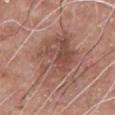  biopsy_status: not biopsied; imaged during a skin examination
  site: front of the torso
  patient:
    sex: male
    age_approx: 60
  lesion_size:
    long_diameter_mm_approx: 8.5
  lighting: white-light
  automated_metrics:
    cielab_L: 50
    cielab_a: 20
    cielab_b: 25
    vs_skin_darker_L: 9.0
    vs_skin_contrast_norm: 6.5
    color_variation_0_10: 5.5
    peripheral_color_asymmetry: 2.0
    nevus_likeness_0_100: 5
    lesion_detection_confidence_0_100: 100
  image:
    source: total-body photography crop
    field_of_view_mm: 15